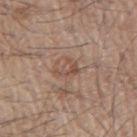Case summary:
– biopsy status · imaged on a skin check; not biopsied
– lighting · white-light
– lesion size · ~3 mm (longest diameter)
– acquisition · total-body-photography crop, ~15 mm field of view
– anatomic site · the right upper arm
– image-analysis metrics · a lesion area of about 3.5 mm² and an eccentricity of roughly 0.65; a mean CIELAB color near L≈50 a*≈19 b*≈27, a lesion–skin lightness drop of about 8, and a normalized lesion–skin contrast near 6; an automated nevus-likeness rating near 0 out of 100 and a lesion-detection confidence of about 100/100
– patient · male, aged approximately 65